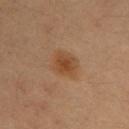follow-up: imaged on a skin check; not biopsied
patient: female, roughly 35 years of age
anatomic site: the right upper arm
tile lighting: cross-polarized illumination
size: about 3.5 mm
image-analysis metrics: a footprint of about 8 mm² and a shape-asymmetry score of about 0.2 (0 = symmetric); roughly 8 lightness units darker than nearby skin
acquisition: ~15 mm tile from a whole-body skin photo